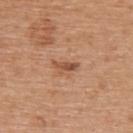Impression:
No biopsy was performed on this lesion — it was imaged during a full skin examination and was not determined to be concerning.
Acquisition and patient details:
Imaged with white-light lighting. Measured at roughly 3 mm in maximum diameter. A female subject in their mid- to late 60s. The lesion-visualizer software estimated an average lesion color of about L≈52 a*≈23 b*≈32 (CIELAB) and a normalized lesion–skin contrast near 7.5. The software also gave border irregularity of about 4 on a 0–10 scale, a within-lesion color-variation index near 1/10, and radial color variation of about 0. The software also gave an automated nevus-likeness rating near 0 out of 100 and a detector confidence of about 100 out of 100 that the crop contains a lesion. A 15 mm close-up tile from a total-body photography series done for melanoma screening. The lesion is located on the upper back.